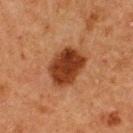The lesion was tiled from a total-body skin photograph and was not biopsied. The recorded lesion diameter is about 5 mm. This is a cross-polarized tile. A female patient aged approximately 50. On the upper back. A close-up tile cropped from a whole-body skin photograph, about 15 mm across.